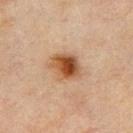size = ~3.5 mm (longest diameter)
image-analysis metrics = roughly 13 lightness units darker than nearby skin and a normalized border contrast of about 11; an automated nevus-likeness rating near 100 out of 100
patient = male, aged 68 to 72
site = the chest
image = ~15 mm crop, total-body skin-cancer survey